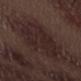The lesion was tiled from a total-body skin photograph and was not biopsied.
The lesion-visualizer software estimated a footprint of about 28 mm², a shape eccentricity near 0.55, and two-axis asymmetry of about 0.25. It also reported border irregularity of about 4 on a 0–10 scale and a color-variation rating of about 3/10. The software also gave a classifier nevus-likeness of about 5/100 and a lesion-detection confidence of about 100/100.
A male patient, aged 68–72.
Imaged with white-light lighting.
Cropped from a total-body skin-imaging series; the visible field is about 15 mm.
Located on the left forearm.